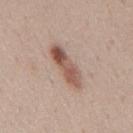Impression: Imaged during a routine full-body skin examination; the lesion was not biopsied and no histopathology is available. Clinical summary: The recorded lesion diameter is about 6 mm. A male subject aged 38 to 42. Located on the mid back. The total-body-photography lesion software estimated a lesion area of about 9 mm² and an outline eccentricity of about 0.95 (0 = round, 1 = elongated). And it measured border irregularity of about 3 on a 0–10 scale, internal color variation of about 7.5 on a 0–10 scale, and peripheral color asymmetry of about 2.5. The analysis additionally found an automated nevus-likeness rating near 80 out of 100 and a lesion-detection confidence of about 100/100. Cropped from a whole-body photographic skin survey; the tile spans about 15 mm.